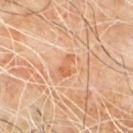Q: Lesion location?
A: the back
Q: What are the patient's age and sex?
A: male, in their 60s
Q: What kind of image is this?
A: ~15 mm crop, total-body skin-cancer survey
Q: How was the tile lit?
A: cross-polarized